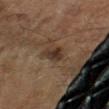Clinical impression: Recorded during total-body skin imaging; not selected for excision or biopsy. Image and clinical context: Longest diameter approximately 2.5 mm. A 15 mm close-up tile from a total-body photography series done for melanoma screening. On the left forearm. An algorithmic analysis of the crop reported a classifier nevus-likeness of about 60/100 and a detector confidence of about 100 out of 100 that the crop contains a lesion. A male subject about 60 years old.